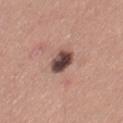Q: Was a biopsy performed?
A: total-body-photography surveillance lesion; no biopsy
Q: Patient demographics?
A: female, about 30 years old
Q: What kind of image is this?
A: ~15 mm tile from a whole-body skin photo
Q: How was the tile lit?
A: white-light
Q: Lesion location?
A: the left thigh
Q: How large is the lesion?
A: ~3.5 mm (longest diameter)
Q: Automated lesion metrics?
A: a lesion area of about 7 mm²; an average lesion color of about L≈45 a*≈19 b*≈21 (CIELAB) and a normalized border contrast of about 13; a border-irregularity index near 1.5/10, a color-variation rating of about 8.5/10, and a peripheral color-asymmetry measure near 3; a classifier nevus-likeness of about 25/100 and a lesion-detection confidence of about 100/100